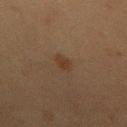The lesion was tiled from a total-body skin photograph and was not biopsied.
A 15 mm close-up tile from a total-body photography series done for melanoma screening.
The subject is a female aged around 50.
This is a cross-polarized tile.
The lesion is on the back.